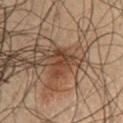| key | value |
|---|---|
| follow-up | no biopsy performed (imaged during a skin exam) |
| automated lesion analysis | a footprint of about 5 mm², a shape eccentricity near 0.65, and a symmetry-axis asymmetry near 0.55 |
| body site | the chest |
| patient | male, aged 48 to 52 |
| tile lighting | cross-polarized |
| acquisition | ~15 mm tile from a whole-body skin photo |
| size | ~3.5 mm (longest diameter) |